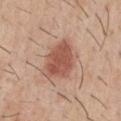Captured during whole-body skin photography for melanoma surveillance; the lesion was not biopsied. A roughly 15 mm field-of-view crop from a total-body skin photograph. The lesion is located on the chest. Captured under white-light illumination. The subject is a male aged 28–32.